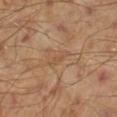Background:
The recorded lesion diameter is about 3 mm. A male patient aged approximately 45. A lesion tile, about 15 mm wide, cut from a 3D total-body photograph. The lesion is on the left lower leg. An algorithmic analysis of the crop reported border irregularity of about 5 on a 0–10 scale, a color-variation rating of about 0/10, and peripheral color asymmetry of about 0. The analysis additionally found a classifier nevus-likeness of about 0/100.